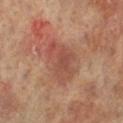Located on the leg.
A 15 mm crop from a total-body photograph taken for skin-cancer surveillance.
The subject is a female in their mid- to late 60s.
An algorithmic analysis of the crop reported an outline eccentricity of about 0.75 (0 = round, 1 = elongated) and two-axis asymmetry of about 0.3. The analysis additionally found a mean CIELAB color near L≈48 a*≈24 b*≈28 and a normalized border contrast of about 6. And it measured a border-irregularity rating of about 3.5/10, internal color variation of about 5 on a 0–10 scale, and peripheral color asymmetry of about 2.